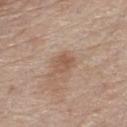Clinical impression: Part of a total-body skin-imaging series; this lesion was reviewed on a skin check and was not flagged for biopsy. Clinical summary: A roughly 15 mm field-of-view crop from a total-body skin photograph. The tile uses white-light illumination. The patient is a male in their 80s. Approximately 3 mm at its widest. On the chest. Automated image analysis of the tile measured an eccentricity of roughly 0.7. The analysis additionally found a normalized lesion–skin contrast near 6. The software also gave an automated nevus-likeness rating near 10 out of 100 and a detector confidence of about 100 out of 100 that the crop contains a lesion.